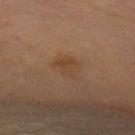The lesion was tiled from a total-body skin photograph and was not biopsied.